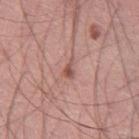From the left thigh. A roughly 15 mm field-of-view crop from a total-body skin photograph. A male subject aged approximately 45. Captured under white-light illumination.